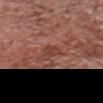notes: catalogued during a skin exam; not biopsied
image-analysis metrics: an average lesion color of about L≈41 a*≈26 b*≈26 (CIELAB), a lesion–skin lightness drop of about 7, and a normalized lesion–skin contrast near 6; border irregularity of about 3 on a 0–10 scale, a within-lesion color-variation index near 1.5/10, and a peripheral color-asymmetry measure near 0.5; an automated nevus-likeness rating near 0 out of 100
lighting: white-light
anatomic site: the head or neck
patient: male, about 65 years old
image: 15 mm crop, total-body photography